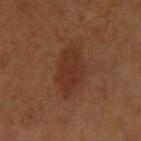| field | value |
|---|---|
| notes | total-body-photography surveillance lesion; no biopsy |
| subject | male, approximately 60 years of age |
| body site | the left upper arm |
| image | ~15 mm crop, total-body skin-cancer survey |
| automated metrics | a footprint of about 12 mm² and a symmetry-axis asymmetry near 0.2; an average lesion color of about L≈29 a*≈21 b*≈27 (CIELAB), a lesion–skin lightness drop of about 6, and a normalized lesion–skin contrast near 6; a border-irregularity rating of about 2.5/10, a color-variation rating of about 2.5/10, and peripheral color asymmetry of about 1; a classifier nevus-likeness of about 50/100 and a lesion-detection confidence of about 100/100 |
| diameter | ~5.5 mm (longest diameter) |
| tile lighting | cross-polarized illumination |